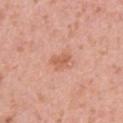Recorded during total-body skin imaging; not selected for excision or biopsy. The patient is a female aged approximately 40. Located on the right upper arm. Cropped from a whole-body photographic skin survey; the tile spans about 15 mm.